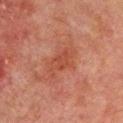{
  "biopsy_status": "not biopsied; imaged during a skin examination",
  "site": "upper back",
  "patient": {
    "sex": "male",
    "age_approx": 65
  },
  "lighting": "cross-polarized",
  "image": {
    "source": "total-body photography crop",
    "field_of_view_mm": 15
  },
  "lesion_size": {
    "long_diameter_mm_approx": 4.5
  }
}